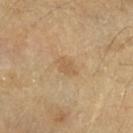biopsy status: catalogued during a skin exam; not biopsied | anatomic site: the arm | tile lighting: cross-polarized illumination | diameter: ≈2.5 mm | image source: 15 mm crop, total-body photography | automated lesion analysis: an area of roughly 3.5 mm²; about 7 CIELAB-L* units darker than the surrounding skin and a lesion-to-skin contrast of about 5 (normalized; higher = more distinct); a nevus-likeness score of about 0/100 and a lesion-detection confidence of about 100/100 | subject: female, aged 58–62.